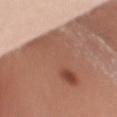Q: What are the patient's age and sex?
A: female, approximately 50 years of age
Q: What is the anatomic site?
A: the left upper arm
Q: What lighting was used for the tile?
A: white-light
Q: What is the imaging modality?
A: 15 mm crop, total-body photography